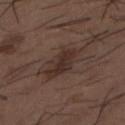workup: catalogued during a skin exam; not biopsied
tile lighting: white-light
patient: male, about 50 years old
diameter: about 7 mm
body site: the back
imaging modality: ~15 mm crop, total-body skin-cancer survey
TBP lesion metrics: an average lesion color of about L≈32 a*≈15 b*≈20 (CIELAB), about 7 CIELAB-L* units darker than the surrounding skin, and a normalized border contrast of about 7; border irregularity of about 4 on a 0–10 scale and a within-lesion color-variation index near 4/10